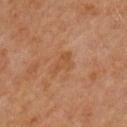  biopsy_status: not biopsied; imaged during a skin examination
  patient:
    sex: male
    age_approx: 65
  image:
    source: total-body photography crop
    field_of_view_mm: 15
  site: arm